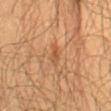Findings:
* biopsy status · no biopsy performed (imaged during a skin exam)
* subject · male, about 60 years old
* diameter · ≈2.5 mm
* tile lighting · cross-polarized illumination
* imaging modality · total-body-photography crop, ~15 mm field of view
* body site · the abdomen
* image-analysis metrics · an area of roughly 3 mm², an outline eccentricity of about 0.9 (0 = round, 1 = elongated), and two-axis asymmetry of about 0.25; a mean CIELAB color near L≈49 a*≈22 b*≈35, roughly 7 lightness units darker than nearby skin, and a lesion-to-skin contrast of about 5.5 (normalized; higher = more distinct); an automated nevus-likeness rating near 5 out of 100 and lesion-presence confidence of about 100/100